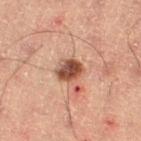workup: total-body-photography surveillance lesion; no biopsy
lesion size: ≈3 mm
imaging modality: 15 mm crop, total-body photography
illumination: cross-polarized illumination
TBP lesion metrics: a border-irregularity index near 2/10, a within-lesion color-variation index near 5/10, and peripheral color asymmetry of about 1.5; a nevus-likeness score of about 95/100 and a detector confidence of about 100 out of 100 that the crop contains a lesion
subject: male, aged approximately 60